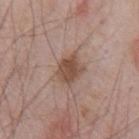Case summary:
– biopsy status: total-body-photography surveillance lesion; no biopsy
– size: ~3.5 mm (longest diameter)
– anatomic site: the chest
– subject: male, aged approximately 70
– image source: ~15 mm crop, total-body skin-cancer survey
– tile lighting: white-light